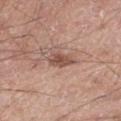- patient: male, aged approximately 60
- automated lesion analysis: a footprint of about 4 mm² and a shape-asymmetry score of about 0.35 (0 = symmetric); a nevus-likeness score of about 60/100
- lighting: white-light illumination
- site: the right thigh
- image: total-body-photography crop, ~15 mm field of view